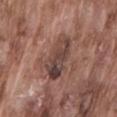workup = catalogued during a skin exam; not biopsied
patient = male, aged approximately 75
image = 15 mm crop, total-body photography
site = the lower back